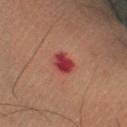biopsy status: catalogued during a skin exam; not biopsied | illumination: cross-polarized | diameter: ≈3 mm | imaging modality: ~15 mm crop, total-body skin-cancer survey | subject: male, about 50 years old | anatomic site: the right lower leg.